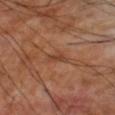  biopsy_status: not biopsied; imaged during a skin examination
  lighting: cross-polarized
  site: right upper arm
  image:
    source: total-body photography crop
    field_of_view_mm: 15
  patient:
    sex: male
    age_approx: 70
  automated_metrics:
    eccentricity: 0.9
    shape_asymmetry: 0.4
    cielab_L: 41
    cielab_a: 22
    cielab_b: 30
    vs_skin_darker_L: 7.0